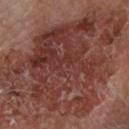Part of a total-body skin-imaging series; this lesion was reviewed on a skin check and was not flagged for biopsy. The tile uses cross-polarized illumination. The subject is a male approximately 65 years of age. An algorithmic analysis of the crop reported a mean CIELAB color near L≈37 a*≈23 b*≈23 and about 9 CIELAB-L* units darker than the surrounding skin. The software also gave a lesion-detection confidence of about 95/100. The lesion is located on the arm. Longest diameter approximately 16 mm. A 15 mm crop from a total-body photograph taken for skin-cancer surveillance.